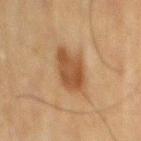Notes:
• notes — imaged on a skin check; not biopsied
• site — the mid back
• lesion diameter — ~5 mm (longest diameter)
• illumination — cross-polarized illumination
• image source — ~15 mm crop, total-body skin-cancer survey
• automated lesion analysis — a lesion color around L≈41 a*≈18 b*≈31 in CIELAB, a lesion–skin lightness drop of about 10, and a normalized border contrast of about 8.5
• patient — male, approximately 70 years of age